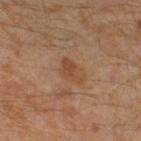  biopsy_status: not biopsied; imaged during a skin examination
  site: left leg
  patient:
    sex: male
    age_approx: 30
  lesion_size:
    long_diameter_mm_approx: 3.5
  image:
    source: total-body photography crop
    field_of_view_mm: 15
  lighting: cross-polarized